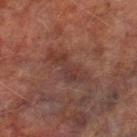Assessment:
Imaged during a routine full-body skin examination; the lesion was not biopsied and no histopathology is available.
Background:
This is a cross-polarized tile. The total-body-photography lesion software estimated a mean CIELAB color near L≈29 a*≈18 b*≈20, a lesion–skin lightness drop of about 5, and a lesion-to-skin contrast of about 5.5 (normalized; higher = more distinct). It also reported a border-irregularity index near 6/10 and a peripheral color-asymmetry measure near 2. And it measured a nevus-likeness score of about 0/100 and a lesion-detection confidence of about 100/100. From the left lower leg. A male subject, approximately 75 years of age. Cropped from a whole-body photographic skin survey; the tile spans about 15 mm.